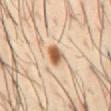Image and clinical context:
Imaged with cross-polarized lighting. The subject is a male aged approximately 40. The lesion is located on the abdomen. The total-body-photography lesion software estimated a mean CIELAB color near L≈59 a*≈20 b*≈35, roughly 16 lightness units darker than nearby skin, and a normalized lesion–skin contrast near 10.5. It also reported a border-irregularity index near 1.5/10. A 15 mm crop from a total-body photograph taken for skin-cancer surveillance.